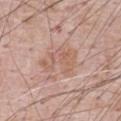{"biopsy_status": "not biopsied; imaged during a skin examination", "lighting": "white-light", "site": "abdomen", "patient": {"sex": "male", "age_approx": 70}, "image": {"source": "total-body photography crop", "field_of_view_mm": 15}, "lesion_size": {"long_diameter_mm_approx": 4.5}, "automated_metrics": {"shape_asymmetry": 0.5, "cielab_L": 59, "cielab_a": 20, "cielab_b": 28, "vs_skin_darker_L": 7.0, "vs_skin_contrast_norm": 5.0, "nevus_likeness_0_100": 0, "lesion_detection_confidence_0_100": 100}}